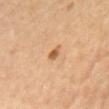Impression:
The lesion was tiled from a total-body skin photograph and was not biopsied.
Clinical summary:
A female patient aged 63–67. The lesion is on the chest. This image is a 15 mm lesion crop taken from a total-body photograph.